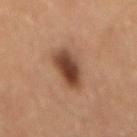The lesion was tiled from a total-body skin photograph and was not biopsied.
The subject is a male aged 58–62.
This is a cross-polarized tile.
A roughly 15 mm field-of-view crop from a total-body skin photograph.
The lesion's longest dimension is about 5 mm.